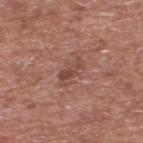Q: Was this lesion biopsied?
A: no biopsy performed (imaged during a skin exam)
Q: What did automated image analysis measure?
A: a lesion area of about 3.5 mm² and an outline eccentricity of about 0.9 (0 = round, 1 = elongated); a border-irregularity rating of about 4/10, internal color variation of about 1 on a 0–10 scale, and a peripheral color-asymmetry measure near 0
Q: Who is the patient?
A: male, approximately 75 years of age
Q: Where on the body is the lesion?
A: the back
Q: How large is the lesion?
A: about 3 mm
Q: What is the imaging modality?
A: ~15 mm crop, total-body skin-cancer survey